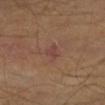Q: Was a biopsy performed?
A: no biopsy performed (imaged during a skin exam)
Q: Where on the body is the lesion?
A: the right lower leg
Q: Patient demographics?
A: male, aged around 40
Q: Illumination type?
A: cross-polarized illumination
Q: What is the imaging modality?
A: ~15 mm tile from a whole-body skin photo